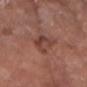Captured during whole-body skin photography for melanoma surveillance; the lesion was not biopsied. From the left forearm. The recorded lesion diameter is about 3.5 mm. The total-body-photography lesion software estimated a footprint of about 6.5 mm² and a shape-asymmetry score of about 0.35 (0 = symmetric). It also reported a lesion–skin lightness drop of about 8 and a normalized lesion–skin contrast near 7. The software also gave a border-irregularity index near 6/10. A roughly 15 mm field-of-view crop from a total-body skin photograph. The patient is a female about 70 years old. The tile uses white-light illumination.